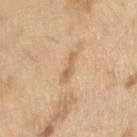Imaged during a routine full-body skin examination; the lesion was not biopsied and no histopathology is available.
From the arm.
A male subject, aged approximately 70.
The tile uses white-light illumination.
A 15 mm crop from a total-body photograph taken for skin-cancer surveillance.